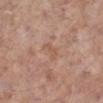notes = imaged on a skin check; not biopsied | patient = female, in their mid- to late 70s | acquisition = ~15 mm crop, total-body skin-cancer survey | lesion diameter = ~2.5 mm (longest diameter) | illumination = white-light illumination | site = the left lower leg.